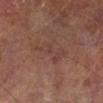Findings:
• notes — catalogued during a skin exam; not biopsied
• automated lesion analysis — a shape eccentricity near 0.9 and two-axis asymmetry of about 0.55; about 5 CIELAB-L* units darker than the surrounding skin and a normalized border contrast of about 4.5; a border-irregularity index near 8/10, internal color variation of about 2 on a 0–10 scale, and peripheral color asymmetry of about 0.5
• imaging modality — ~15 mm crop, total-body skin-cancer survey
• subject — male, approximately 65 years of age
• diameter — ≈5 mm
• location — the left lower leg